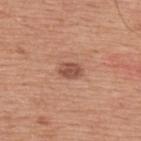• workup · imaged on a skin check; not biopsied
• tile lighting · white-light illumination
• TBP lesion metrics · a shape eccentricity near 0.75 and two-axis asymmetry of about 0.2; a lesion color around L≈51 a*≈24 b*≈29 in CIELAB and a normalized border contrast of about 8
• body site · the upper back
• diameter · ~2.5 mm (longest diameter)
• subject · male, aged approximately 75
• image · ~15 mm tile from a whole-body skin photo